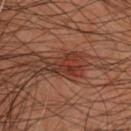Notes:
- notes · total-body-photography surveillance lesion; no biopsy
- imaging modality · total-body-photography crop, ~15 mm field of view
- diameter · about 4 mm
- TBP lesion metrics · an outline eccentricity of about 0.8 (0 = round, 1 = elongated) and a shape-asymmetry score of about 0.6 (0 = symmetric); a mean CIELAB color near L≈33 a*≈23 b*≈27, roughly 9 lightness units darker than nearby skin, and a normalized lesion–skin contrast near 8; a border-irregularity rating of about 7.5/10 and a within-lesion color-variation index near 5.5/10
- location · the upper back
- patient · male, approximately 45 years of age